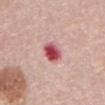Clinical impression: Recorded during total-body skin imaging; not selected for excision or biopsy. Context: This is a white-light tile. An algorithmic analysis of the crop reported a lesion color around L≈53 a*≈36 b*≈21 in CIELAB, about 18 CIELAB-L* units darker than the surrounding skin, and a normalized lesion–skin contrast near 11.5. The software also gave border irregularity of about 2 on a 0–10 scale, internal color variation of about 7 on a 0–10 scale, and a peripheral color-asymmetry measure near 2. A female patient, aged 63–67. A close-up tile cropped from a whole-body skin photograph, about 15 mm across. The lesion is on the abdomen.